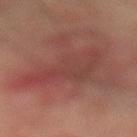  lighting: cross-polarized
  site: leg
  patient:
    sex: male
    age_approx: 75
  image:
    source: total-body photography crop
    field_of_view_mm: 15
  lesion_size:
    long_diameter_mm_approx: 8.0
  automated_metrics:
    cielab_L: 35
    cielab_a: 22
    cielab_b: 20
    nevus_likeness_0_100: 0
    lesion_detection_confidence_0_100: 50